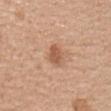Assessment:
No biopsy was performed on this lesion — it was imaged during a full skin examination and was not determined to be concerning.
Clinical summary:
Cropped from a whole-body photographic skin survey; the tile spans about 15 mm. The lesion is located on the back. This is a white-light tile. Approximately 2.5 mm at its widest. A female subject aged approximately 25.